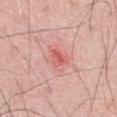workup: catalogued during a skin exam; not biopsied | acquisition: ~15 mm crop, total-body skin-cancer survey | anatomic site: the front of the torso | subject: male, about 60 years old.